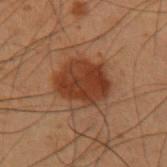| feature | finding |
|---|---|
| automated metrics | a lesion color around L≈31 a*≈20 b*≈27 in CIELAB, about 9 CIELAB-L* units darker than the surrounding skin, and a normalized border contrast of about 9; a border-irregularity rating of about 2/10, a color-variation rating of about 4/10, and peripheral color asymmetry of about 1.5 |
| subject | male, roughly 55 years of age |
| site | the arm |
| imaging modality | ~15 mm crop, total-body skin-cancer survey |
| illumination | cross-polarized |
| diameter | ~5.5 mm (longest diameter) |